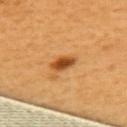Impression: Captured during whole-body skin photography for melanoma surveillance; the lesion was not biopsied. Clinical summary: The patient is a female in their mid- to late 50s. The total-body-photography lesion software estimated a footprint of about 4.5 mm², an outline eccentricity of about 0.85 (0 = round, 1 = elongated), and two-axis asymmetry of about 0.2. On the upper back. A close-up tile cropped from a whole-body skin photograph, about 15 mm across. The tile uses cross-polarized illumination.